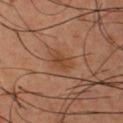{"biopsy_status": "not biopsied; imaged during a skin examination", "automated_metrics": {"area_mm2_approx": 4.5, "eccentricity": 0.8, "shape_asymmetry": 0.2}, "site": "front of the torso", "patient": {"sex": "male", "age_approx": 40}, "lesion_size": {"long_diameter_mm_approx": 3.0}, "lighting": "cross-polarized", "image": {"source": "total-body photography crop", "field_of_view_mm": 15}}